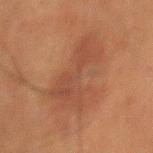| feature | finding |
|---|---|
| follow-up | catalogued during a skin exam; not biopsied |
| anatomic site | the mid back |
| patient | male, aged 48–52 |
| image source | ~15 mm tile from a whole-body skin photo |
| lighting | cross-polarized |